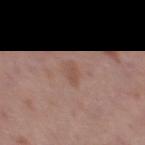A female patient, aged 38–42. The total-body-photography lesion software estimated a lesion area of about 3 mm², a shape eccentricity near 0.8, and a symmetry-axis asymmetry near 0.35. About 2.5 mm across. From the left thigh. A region of skin cropped from a whole-body photographic capture, roughly 15 mm wide. The tile uses white-light illumination.